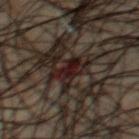No biopsy was performed on this lesion — it was imaged during a full skin examination and was not determined to be concerning.
About 3.5 mm across.
This is a cross-polarized tile.
Located on the chest.
A 15 mm close-up tile from a total-body photography series done for melanoma screening.
A male subject in their 50s.
Automated tile analysis of the lesion measured a lesion color around L≈13 a*≈13 b*≈12 in CIELAB. It also reported a border-irregularity index near 6/10, internal color variation of about 3.5 on a 0–10 scale, and radial color variation of about 1. The analysis additionally found a classifier nevus-likeness of about 0/100.